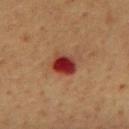Context: The lesion is on the mid back. The lesion-visualizer software estimated a mean CIELAB color near L≈32 a*≈30 b*≈27, a lesion–skin lightness drop of about 15, and a normalized lesion–skin contrast near 13. The software also gave a classifier nevus-likeness of about 0/100 and a detector confidence of about 100 out of 100 that the crop contains a lesion. Cropped from a total-body skin-imaging series; the visible field is about 15 mm. A male patient about 60 years old. Longest diameter approximately 3 mm.